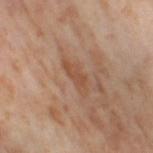Impression:
Recorded during total-body skin imaging; not selected for excision or biopsy.
Context:
The total-body-photography lesion software estimated a lesion area of about 5 mm², an eccentricity of roughly 0.95, and a symmetry-axis asymmetry near 0.4. The analysis additionally found a mean CIELAB color near L≈51 a*≈21 b*≈32, roughly 7 lightness units darker than nearby skin, and a normalized border contrast of about 6. The analysis additionally found a border-irregularity index near 4.5/10 and a within-lesion color-variation index near 1.5/10. The software also gave a nevus-likeness score of about 0/100 and lesion-presence confidence of about 60/100. Longest diameter approximately 4.5 mm. The lesion is located on the right thigh. A close-up tile cropped from a whole-body skin photograph, about 15 mm across. Captured under cross-polarized illumination. A female subject, in their mid-50s.